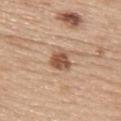Findings:
* follow-up · no biopsy performed (imaged during a skin exam)
* image source · total-body-photography crop, ~15 mm field of view
* subject · female, approximately 65 years of age
* body site · the back